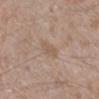The lesion was photographed on a routine skin check and not biopsied; there is no pathology result. The lesion is on the leg. A male subject aged 43 to 47. Cropped from a whole-body photographic skin survey; the tile spans about 15 mm. Imaged with white-light lighting. An algorithmic analysis of the crop reported an average lesion color of about L≈56 a*≈16 b*≈28 (CIELAB), about 6 CIELAB-L* units darker than the surrounding skin, and a normalized lesion–skin contrast near 5. And it measured internal color variation of about 1.5 on a 0–10 scale and a peripheral color-asymmetry measure near 0.5. Measured at roughly 3 mm in maximum diameter.